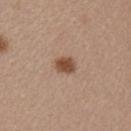Q: Is there a histopathology result?
A: imaged on a skin check; not biopsied
Q: What did automated image analysis measure?
A: a mean CIELAB color near L≈50 a*≈19 b*≈29, roughly 12 lightness units darker than nearby skin, and a normalized lesion–skin contrast near 9; a color-variation rating of about 2.5/10 and peripheral color asymmetry of about 0.5; an automated nevus-likeness rating near 100 out of 100 and a detector confidence of about 100 out of 100 that the crop contains a lesion
Q: Patient demographics?
A: female, aged approximately 45
Q: Lesion size?
A: about 2.5 mm
Q: What is the anatomic site?
A: the left upper arm
Q: What kind of image is this?
A: ~15 mm tile from a whole-body skin photo